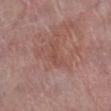Q: What is the lesion's diameter?
A: about 3.5 mm
Q: Automated lesion metrics?
A: a lesion color around L≈49 a*≈22 b*≈26 in CIELAB, about 5 CIELAB-L* units darker than the surrounding skin, and a normalized border contrast of about 4; an automated nevus-likeness rating near 0 out of 100
Q: How was this image acquired?
A: ~15 mm tile from a whole-body skin photo
Q: Illumination type?
A: white-light illumination
Q: Lesion location?
A: the leg
Q: Who is the patient?
A: female, aged 58–62